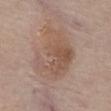Q: Was this lesion biopsied?
A: no biopsy performed (imaged during a skin exam)
Q: Automated lesion metrics?
A: a within-lesion color-variation index near 4.5/10 and peripheral color asymmetry of about 1.5; a classifier nevus-likeness of about 30/100 and a lesion-detection confidence of about 100/100
Q: What is the imaging modality?
A: ~15 mm crop, total-body skin-cancer survey
Q: Where on the body is the lesion?
A: the abdomen
Q: Patient demographics?
A: male, aged approximately 85 or older
Q: How was the tile lit?
A: white-light
Q: How large is the lesion?
A: ≈7 mm